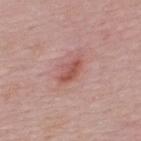{"biopsy_status": "not biopsied; imaged during a skin examination", "image": {"source": "total-body photography crop", "field_of_view_mm": 15}, "lesion_size": {"long_diameter_mm_approx": 3.0}, "patient": {"sex": "male", "age_approx": 50}, "site": "upper back", "lighting": "white-light"}